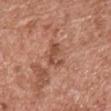No biopsy was performed on this lesion — it was imaged during a full skin examination and was not determined to be concerning. The lesion is located on the chest. Imaged with white-light lighting. A lesion tile, about 15 mm wide, cut from a 3D total-body photograph. A male patient aged around 70. The lesion's longest dimension is about 3 mm.